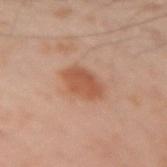Q: Is there a histopathology result?
A: imaged on a skin check; not biopsied
Q: What is the imaging modality?
A: ~15 mm tile from a whole-body skin photo
Q: What is the anatomic site?
A: the arm
Q: How was the tile lit?
A: cross-polarized illumination
Q: What are the patient's age and sex?
A: male, aged 48–52
Q: Lesion size?
A: about 4 mm